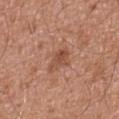Captured during whole-body skin photography for melanoma surveillance; the lesion was not biopsied.
Cropped from a total-body skin-imaging series; the visible field is about 15 mm.
The lesion's longest dimension is about 3.5 mm.
The tile uses white-light illumination.
A male patient, approximately 75 years of age.
An algorithmic analysis of the crop reported a within-lesion color-variation index near 3.5/10 and radial color variation of about 1.5. The analysis additionally found a lesion-detection confidence of about 100/100.
On the abdomen.